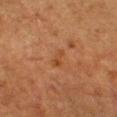Clinical impression:
No biopsy was performed on this lesion — it was imaged during a full skin examination and was not determined to be concerning.
Image and clinical context:
Cropped from a total-body skin-imaging series; the visible field is about 15 mm. This is a cross-polarized tile. A female patient, aged 53–57. Measured at roughly 2.5 mm in maximum diameter. The lesion is located on the head or neck. The total-body-photography lesion software estimated a mean CIELAB color near L≈38 a*≈21 b*≈33, about 5 CIELAB-L* units darker than the surrounding skin, and a normalized border contrast of about 5.5. The software also gave lesion-presence confidence of about 100/100.